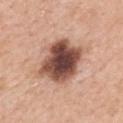Captured during whole-body skin photography for melanoma surveillance; the lesion was not biopsied.
The subject is a female aged 38 to 42.
The total-body-photography lesion software estimated an area of roughly 21 mm², an eccentricity of roughly 0.45, and a symmetry-axis asymmetry near 0.15. And it measured an automated nevus-likeness rating near 55 out of 100 and a detector confidence of about 100 out of 100 that the crop contains a lesion.
The recorded lesion diameter is about 5 mm.
The lesion is located on the mid back.
A close-up tile cropped from a whole-body skin photograph, about 15 mm across.
Imaged with white-light lighting.